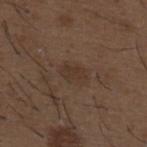Q: Was this lesion biopsied?
A: total-body-photography surveillance lesion; no biopsy
Q: How was the tile lit?
A: white-light illumination
Q: What kind of image is this?
A: total-body-photography crop, ~15 mm field of view
Q: Who is the patient?
A: male, aged 48 to 52
Q: Lesion location?
A: the upper back
Q: What is the lesion's diameter?
A: ~3 mm (longest diameter)